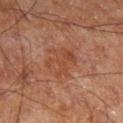Clinical impression: Part of a total-body skin-imaging series; this lesion was reviewed on a skin check and was not flagged for biopsy. Context: From the right lower leg. The tile uses cross-polarized illumination. Approximately 4.5 mm at its widest. A lesion tile, about 15 mm wide, cut from a 3D total-body photograph. An algorithmic analysis of the crop reported a lesion color around L≈45 a*≈24 b*≈32 in CIELAB and a lesion–skin lightness drop of about 6. And it measured a border-irregularity rating of about 5.5/10, a within-lesion color-variation index near 3.5/10, and radial color variation of about 1. A male patient, in their 60s.